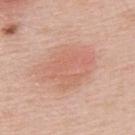Imaged during a routine full-body skin examination; the lesion was not biopsied and no histopathology is available. This is a white-light tile. Measured at roughly 7 mm in maximum diameter. A male patient, about 55 years old. A roughly 15 mm field-of-view crop from a total-body skin photograph. From the upper back.